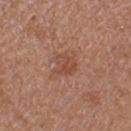Clinical summary: A female subject approximately 40 years of age. A roughly 15 mm field-of-view crop from a total-body skin photograph. The total-body-photography lesion software estimated a shape eccentricity near 0.5 and a symmetry-axis asymmetry near 0.3. Captured under white-light illumination. The lesion's longest dimension is about 3 mm. The lesion is on the leg.